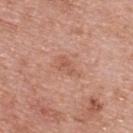Q: Was this lesion biopsied?
A: catalogued during a skin exam; not biopsied
Q: How was this image acquired?
A: 15 mm crop, total-body photography
Q: How was the tile lit?
A: white-light illumination
Q: Patient demographics?
A: female, about 60 years old
Q: Lesion location?
A: the upper back